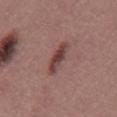workup = total-body-photography surveillance lesion; no biopsy
acquisition = ~15 mm crop, total-body skin-cancer survey
diameter = ≈4 mm
illumination = white-light illumination
site = the back
image-analysis metrics = an area of roughly 6.5 mm², a shape eccentricity near 0.9, and a symmetry-axis asymmetry near 0.25; a mean CIELAB color near L≈42 a*≈22 b*≈21, a lesion–skin lightness drop of about 11, and a normalized lesion–skin contrast near 9; border irregularity of about 3 on a 0–10 scale, a within-lesion color-variation index near 5/10, and a peripheral color-asymmetry measure near 1.5; a classifier nevus-likeness of about 65/100 and a detector confidence of about 100 out of 100 that the crop contains a lesion
patient = male, aged around 40